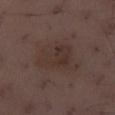Impression:
Part of a total-body skin-imaging series; this lesion was reviewed on a skin check and was not flagged for biopsy.
Image and clinical context:
A 15 mm close-up tile from a total-body photography series done for melanoma screening. The patient is a male aged around 40. Located on the right thigh. Approximately 5.5 mm at its widest. Automated tile analysis of the lesion measured a symmetry-axis asymmetry near 0.3. It also reported an average lesion color of about L≈32 a*≈14 b*≈19 (CIELAB) and a normalized border contrast of about 6.